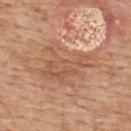Assessment: Captured during whole-body skin photography for melanoma surveillance; the lesion was not biopsied. Acquisition and patient details: The tile uses white-light illumination. Located on the back. Approximately 6.5 mm at its widest. The total-body-photography lesion software estimated a lesion area of about 20 mm² and an outline eccentricity of about 0.7 (0 = round, 1 = elongated). The analysis additionally found border irregularity of about 6.5 on a 0–10 scale, a within-lesion color-variation index near 4/10, and radial color variation of about 1.5. A close-up tile cropped from a whole-body skin photograph, about 15 mm across. A male subject about 60 years old.